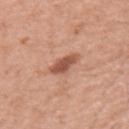Findings:
• notes: imaged on a skin check; not biopsied